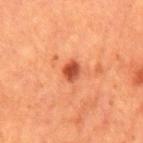A male subject aged approximately 65. A close-up tile cropped from a whole-body skin photograph, about 15 mm across. The recorded lesion diameter is about 2.5 mm. On the mid back. The lesion-visualizer software estimated an area of roughly 3.5 mm², an eccentricity of roughly 0.7, and a shape-asymmetry score of about 0.2 (0 = symmetric).